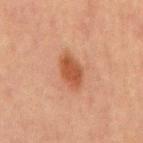<case>
<automated_metrics>
  <vs_skin_darker_L>9.0</vs_skin_darker_L>
  <vs_skin_contrast_norm>8.5</vs_skin_contrast_norm>
  <border_irregularity_0_10>1.5</border_irregularity_0_10>
  <color_variation_0_10>2.0</color_variation_0_10>
</automated_metrics>
<patient>
  <sex>male</sex>
  <age_approx>65</age_approx>
</patient>
<site>abdomen</site>
<lighting>cross-polarized</lighting>
<image>
  <source>total-body photography crop</source>
  <field_of_view_mm>15</field_of_view_mm>
</image>
</case>